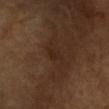| key | value |
|---|---|
| biopsy status | no biopsy performed (imaged during a skin exam) |
| acquisition | ~15 mm crop, total-body skin-cancer survey |
| location | the chest |
| subject | female, in their mid- to late 50s |